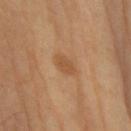| field | value |
|---|---|
| biopsy status | total-body-photography surveillance lesion; no biopsy |
| image-analysis metrics | a lesion area of about 4 mm², a shape eccentricity near 0.7, and a symmetry-axis asymmetry near 0.25; an average lesion color of about L≈53 a*≈21 b*≈37 (CIELAB), roughly 8 lightness units darker than nearby skin, and a normalized lesion–skin contrast near 6; an automated nevus-likeness rating near 0 out of 100 |
| patient | female, in their 70s |
| location | the right upper arm |
| imaging modality | ~15 mm tile from a whole-body skin photo |